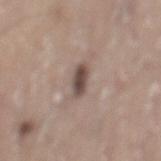No biopsy was performed on this lesion — it was imaged during a full skin examination and was not determined to be concerning. About 3 mm across. A male subject aged 73–77. This image is a 15 mm lesion crop taken from a total-body photograph. The lesion is located on the mid back. The lesion-visualizer software estimated border irregularity of about 2 on a 0–10 scale and internal color variation of about 3.5 on a 0–10 scale. And it measured a lesion-detection confidence of about 100/100.